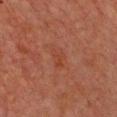Assessment:
No biopsy was performed on this lesion — it was imaged during a full skin examination and was not determined to be concerning.
Image and clinical context:
A female patient in their 70s. Approximately 2.5 mm at its widest. The lesion is on the chest. A lesion tile, about 15 mm wide, cut from a 3D total-body photograph. This is a cross-polarized tile.